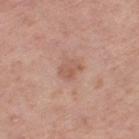notes — imaged on a skin check; not biopsied
body site — the right thigh
patient — male, aged approximately 75
tile lighting — white-light
imaging modality — ~15 mm tile from a whole-body skin photo
size — ~2.5 mm (longest diameter)